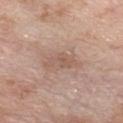Q: Was this lesion biopsied?
A: total-body-photography surveillance lesion; no biopsy
Q: What is the lesion's diameter?
A: ~4 mm (longest diameter)
Q: How was the tile lit?
A: white-light illumination
Q: What are the patient's age and sex?
A: male, aged approximately 60
Q: Lesion location?
A: the chest
Q: What kind of image is this?
A: 15 mm crop, total-body photography